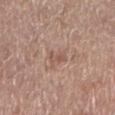Part of a total-body skin-imaging series; this lesion was reviewed on a skin check and was not flagged for biopsy.
A male patient, about 70 years old.
Captured under white-light illumination.
The lesion is on the left lower leg.
About 2.5 mm across.
A 15 mm close-up tile from a total-body photography series done for melanoma screening.